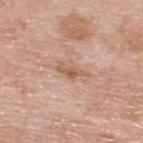| feature | finding |
|---|---|
| lesion size | ≈3.5 mm |
| image-analysis metrics | an area of roughly 4 mm² and an outline eccentricity of about 0.9 (0 = round, 1 = elongated) |
| subject | male, in their 60s |
| lighting | white-light illumination |
| image | ~15 mm tile from a whole-body skin photo |
| anatomic site | the upper back |